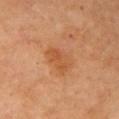{
  "biopsy_status": "not biopsied; imaged during a skin examination",
  "patient": {
    "sex": "female",
    "age_approx": 70
  },
  "automated_metrics": {
    "area_mm2_approx": 8.0,
    "eccentricity": 0.75,
    "shape_asymmetry": 0.25
  },
  "site": "arm",
  "lesion_size": {
    "long_diameter_mm_approx": 4.0
  },
  "lighting": "cross-polarized",
  "image": {
    "source": "total-body photography crop",
    "field_of_view_mm": 15
  }
}